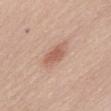  biopsy_status: not biopsied; imaged during a skin examination
  image:
    source: total-body photography crop
    field_of_view_mm: 15
  site: abdomen
  patient:
    sex: female
    age_approx: 50